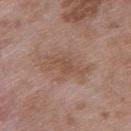Recorded during total-body skin imaging; not selected for excision or biopsy.
The lesion is on the upper back.
The tile uses white-light illumination.
Measured at roughly 5 mm in maximum diameter.
A close-up tile cropped from a whole-body skin photograph, about 15 mm across.
A male patient, aged approximately 50.